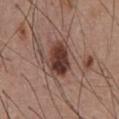workup = no biopsy performed (imaged during a skin exam) | anatomic site = the chest | lighting = white-light | subject = male, in their mid-50s | lesion size = ≈4 mm | automated metrics = roughly 15 lightness units darker than nearby skin; a border-irregularity rating of about 2.5/10, a color-variation rating of about 5/10, and peripheral color asymmetry of about 1.5; lesion-presence confidence of about 100/100 | acquisition = ~15 mm tile from a whole-body skin photo.